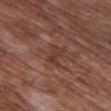| key | value |
|---|---|
| biopsy status | no biopsy performed (imaged during a skin exam) |
| image-analysis metrics | an area of roughly 4.5 mm², an outline eccentricity of about 0.75 (0 = round, 1 = elongated), and a shape-asymmetry score of about 0.3 (0 = symmetric); an average lesion color of about L≈37 a*≈20 b*≈24 (CIELAB), roughly 7 lightness units darker than nearby skin, and a lesion-to-skin contrast of about 6 (normalized; higher = more distinct); internal color variation of about 3 on a 0–10 scale and a peripheral color-asymmetry measure near 1 |
| body site | the chest |
| subject | female, aged approximately 80 |
| image | 15 mm crop, total-body photography |
| diameter | ≈2.5 mm |